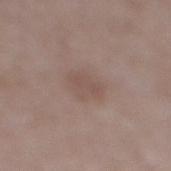workup = no biopsy performed (imaged during a skin exam); image source = ~15 mm crop, total-body skin-cancer survey; anatomic site = the right lower leg; lighting = white-light illumination; subject = male, about 65 years old.